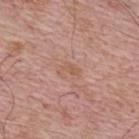The lesion was tiled from a total-body skin photograph and was not biopsied.
Approximately 2.5 mm at its widest.
A lesion tile, about 15 mm wide, cut from a 3D total-body photograph.
The subject is a male aged around 65.
The tile uses white-light illumination.
The lesion is located on the back.